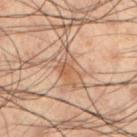Assessment:
Part of a total-body skin-imaging series; this lesion was reviewed on a skin check and was not flagged for biopsy.
Image and clinical context:
The patient is a male about 50 years old. From the left lower leg. Cropped from a whole-body photographic skin survey; the tile spans about 15 mm. About 4.5 mm across. Captured under cross-polarized illumination.